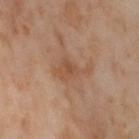workup = total-body-photography surveillance lesion; no biopsy | image source = ~15 mm crop, total-body skin-cancer survey | patient = female, in their mid- to late 50s | site = the right thigh.